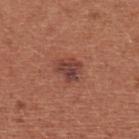Recorded during total-body skin imaging; not selected for excision or biopsy. A region of skin cropped from a whole-body photographic capture, roughly 15 mm wide. The lesion is on the front of the torso. The recorded lesion diameter is about 3 mm. The patient is a female in their mid- to late 30s. An algorithmic analysis of the crop reported a mean CIELAB color near L≈41 a*≈24 b*≈25, about 10 CIELAB-L* units darker than the surrounding skin, and a normalized lesion–skin contrast near 9. And it measured a border-irregularity index near 3/10, a color-variation rating of about 4.5/10, and a peripheral color-asymmetry measure near 1.5. The software also gave an automated nevus-likeness rating near 20 out of 100 and lesion-presence confidence of about 100/100.